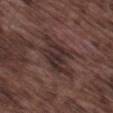Assessment: Recorded during total-body skin imaging; not selected for excision or biopsy. Context: Longest diameter approximately 7 mm. Automated tile analysis of the lesion measured a within-lesion color-variation index near 4.5/10 and radial color variation of about 1.5. And it measured a classifier nevus-likeness of about 0/100 and lesion-presence confidence of about 50/100. A male patient, approximately 75 years of age. Cropped from a whole-body photographic skin survey; the tile spans about 15 mm. The lesion is located on the leg. Captured under white-light illumination.